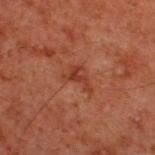automated_metrics:
  cielab_L: 30
  cielab_a: 23
  cielab_b: 27
  vs_skin_contrast_norm: 6.5
  border_irregularity_0_10: 7.0
  color_variation_0_10: 2.5
  peripheral_color_asymmetry: 1.0
image:
  source: total-body photography crop
  field_of_view_mm: 15
patient:
  sex: male
  age_approx: 60
site: upper back